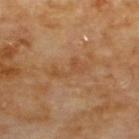The lesion was photographed on a routine skin check and not biopsied; there is no pathology result.
Imaged with cross-polarized lighting.
Measured at roughly 4.5 mm in maximum diameter.
Automated tile analysis of the lesion measured a lesion area of about 5.5 mm² and a shape-asymmetry score of about 0.55 (0 = symmetric).
The lesion is on the upper back.
A male patient, aged around 70.
A close-up tile cropped from a whole-body skin photograph, about 15 mm across.